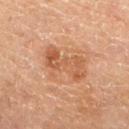site = the left lower leg
diameter = ~6 mm (longest diameter)
subject = female, aged around 55
illumination = cross-polarized illumination
image = total-body-photography crop, ~15 mm field of view